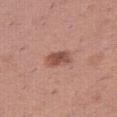The lesion was photographed on a routine skin check and not biopsied; there is no pathology result.
Captured under white-light illumination.
Cropped from a whole-body photographic skin survey; the tile spans about 15 mm.
A female subject, aged 38 to 42.
About 3 mm across.
The lesion is on the right thigh.